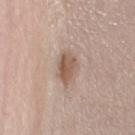{
  "biopsy_status": "not biopsied; imaged during a skin examination",
  "lighting": "white-light",
  "patient": {
    "sex": "female",
    "age_approx": 60
  },
  "image": {
    "source": "total-body photography crop",
    "field_of_view_mm": 15
  },
  "site": "leg"
}